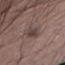Assessment: The lesion was tiled from a total-body skin photograph and was not biopsied. Image and clinical context: A male subject, aged 53 to 57. Automated tile analysis of the lesion measured a footprint of about 7.5 mm² and a symmetry-axis asymmetry near 0.25. The software also gave an average lesion color of about L≈42 a*≈14 b*≈18 (CIELAB), about 9 CIELAB-L* units darker than the surrounding skin, and a normalized lesion–skin contrast near 7.5. From the right thigh. About 5.5 mm across. Imaged with white-light lighting. A region of skin cropped from a whole-body photographic capture, roughly 15 mm wide.